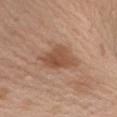Assessment:
Imaged during a routine full-body skin examination; the lesion was not biopsied and no histopathology is available.
Clinical summary:
The tile uses white-light illumination. The lesion-visualizer software estimated a mean CIELAB color near L≈51 a*≈21 b*≈31, roughly 10 lightness units darker than nearby skin, and a normalized border contrast of about 7.5. Cropped from a whole-body photographic skin survey; the tile spans about 15 mm. The subject is a male roughly 55 years of age. From the right upper arm. Measured at roughly 6 mm in maximum diameter.